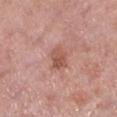Findings:
- notes: total-body-photography surveillance lesion; no biopsy
- body site: the leg
- image source: 15 mm crop, total-body photography
- patient: female, aged 48 to 52